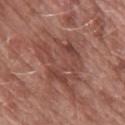| feature | finding |
|---|---|
| patient | female, in their 60s |
| site | the arm |
| image source | 15 mm crop, total-body photography |